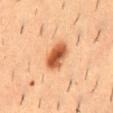Imaged during a routine full-body skin examination; the lesion was not biopsied and no histopathology is available.
A lesion tile, about 15 mm wide, cut from a 3D total-body photograph.
The subject is a male roughly 55 years of age.
On the mid back.